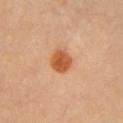Clinical impression:
No biopsy was performed on this lesion — it was imaged during a full skin examination and was not determined to be concerning.
Context:
On the front of the torso. The recorded lesion diameter is about 3 mm. A female subject, in their 60s. A 15 mm close-up tile from a total-body photography series done for melanoma screening. Automated tile analysis of the lesion measured an average lesion color of about L≈57 a*≈28 b*≈41 (CIELAB), a lesion–skin lightness drop of about 13, and a lesion-to-skin contrast of about 9.5 (normalized; higher = more distinct). The software also gave border irregularity of about 1.5 on a 0–10 scale, a color-variation rating of about 4/10, and peripheral color asymmetry of about 1.5. And it measured a classifier nevus-likeness of about 100/100 and lesion-presence confidence of about 100/100. Imaged with cross-polarized lighting.